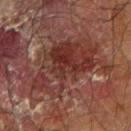The lesion was tiled from a total-body skin photograph and was not biopsied.
A male patient, approximately 70 years of age.
A roughly 15 mm field-of-view crop from a total-body skin photograph.
The lesion is on the left forearm.